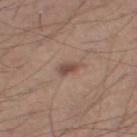Q: Was a biopsy performed?
A: imaged on a skin check; not biopsied
Q: What did automated image analysis measure?
A: a border-irregularity index near 2.5/10 and a color-variation rating of about 1/10; lesion-presence confidence of about 100/100
Q: How large is the lesion?
A: ≈2.5 mm
Q: What is the anatomic site?
A: the right lower leg
Q: What is the imaging modality?
A: ~15 mm tile from a whole-body skin photo
Q: Patient demographics?
A: male, aged 38–42